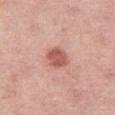Q: How was the tile lit?
A: white-light
Q: What did automated image analysis measure?
A: an area of roughly 6.5 mm², an outline eccentricity of about 0.2 (0 = round, 1 = elongated), and a shape-asymmetry score of about 0.15 (0 = symmetric); a lesion color around L≈57 a*≈27 b*≈28 in CIELAB, about 12 CIELAB-L* units darker than the surrounding skin, and a normalized border contrast of about 8; border irregularity of about 1.5 on a 0–10 scale, a within-lesion color-variation index near 2/10, and peripheral color asymmetry of about 1
Q: Where on the body is the lesion?
A: the right thigh
Q: What kind of image is this?
A: 15 mm crop, total-body photography
Q: Who is the patient?
A: female, aged 43–47
Q: What is the lesion's diameter?
A: about 3 mm